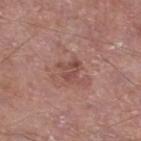biopsy status = no biopsy performed (imaged during a skin exam) | subject = male, in their mid- to late 50s | automated metrics = a lesion area of about 5 mm²; a nevus-likeness score of about 0/100 and lesion-presence confidence of about 100/100 | site = the left lower leg | lesion size = about 3 mm | image = ~15 mm tile from a whole-body skin photo.